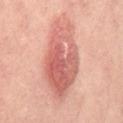- biopsy status — total-body-photography surveillance lesion; no biopsy
- patient — female, aged around 50
- image source — ~15 mm tile from a whole-body skin photo
- tile lighting — cross-polarized illumination
- location — the abdomen